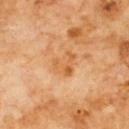biopsy status: imaged on a skin check; not biopsied
location: the chest
image source: 15 mm crop, total-body photography
lesion diameter: ≈3.5 mm
subject: male, aged approximately 60
lighting: cross-polarized